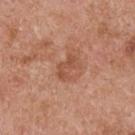{"biopsy_status": "not biopsied; imaged during a skin examination", "site": "upper back", "patient": {"sex": "male", "age_approx": 55}, "lesion_size": {"long_diameter_mm_approx": 3.0}, "image": {"source": "total-body photography crop", "field_of_view_mm": 15}, "automated_metrics": {"area_mm2_approx": 5.0, "eccentricity": 0.8, "shape_asymmetry": 0.4, "border_irregularity_0_10": 5.0, "color_variation_0_10": 2.0, "peripheral_color_asymmetry": 0.5}}